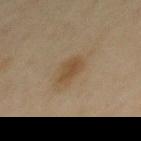Part of a total-body skin-imaging series; this lesion was reviewed on a skin check and was not flagged for biopsy. The recorded lesion diameter is about 3 mm. Cropped from a whole-body photographic skin survey; the tile spans about 15 mm. A female patient, about 45 years old. On the upper back.